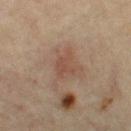The lesion's longest dimension is about 3 mm. This image is a 15 mm lesion crop taken from a total-body photograph. The tile uses cross-polarized illumination. A female subject aged approximately 65. Located on the left thigh.